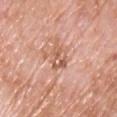Q: Is there a histopathology result?
A: no biopsy performed (imaged during a skin exam)
Q: What are the patient's age and sex?
A: male, about 60 years old
Q: How was this image acquired?
A: ~15 mm tile from a whole-body skin photo
Q: Where on the body is the lesion?
A: the chest